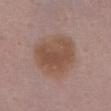workup: total-body-photography surveillance lesion; no biopsy
site: the chest
patient: female, approximately 50 years of age
imaging modality: ~15 mm crop, total-body skin-cancer survey